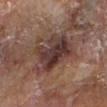Assessment:
Imaged during a routine full-body skin examination; the lesion was not biopsied and no histopathology is available.
Background:
Longest diameter approximately 6.5 mm. A male subject, in their mid- to late 80s or older. Cropped from a whole-body photographic skin survey; the tile spans about 15 mm. Automated tile analysis of the lesion measured a lesion area of about 21 mm² and a symmetry-axis asymmetry near 0.3. It also reported border irregularity of about 4.5 on a 0–10 scale, a color-variation rating of about 7.5/10, and a peripheral color-asymmetry measure near 2.5. From the right forearm. The tile uses cross-polarized illumination.